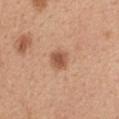Clinical summary:
Imaged with white-light lighting. The patient is a female aged around 45. Automated tile analysis of the lesion measured a border-irregularity index near 1.5/10 and a peripheral color-asymmetry measure near 0.5. The software also gave an automated nevus-likeness rating near 90 out of 100. A 15 mm crop from a total-body photograph taken for skin-cancer surveillance. The recorded lesion diameter is about 2.5 mm. The lesion is located on the upper back.